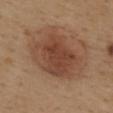The recorded lesion diameter is about 7 mm. An algorithmic analysis of the crop reported a shape eccentricity near 0.65 and a shape-asymmetry score of about 0.2 (0 = symmetric). And it measured an average lesion color of about L≈44 a*≈21 b*≈29 (CIELAB) and a normalized border contrast of about 7.5. A 15 mm close-up extracted from a 3D total-body photography capture. From the upper back. The patient is a female aged around 40.